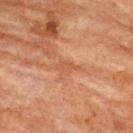workup = imaged on a skin check; not biopsied
imaging modality = total-body-photography crop, ~15 mm field of view
subject = female, about 80 years old
body site = the upper back
image-analysis metrics = a color-variation rating of about 1/10 and radial color variation of about 0.5; a classifier nevus-likeness of about 0/100 and a detector confidence of about 55 out of 100 that the crop contains a lesion
tile lighting = cross-polarized illumination